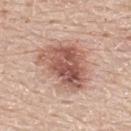Captured during whole-body skin photography for melanoma surveillance; the lesion was not biopsied.
Cropped from a total-body skin-imaging series; the visible field is about 15 mm.
The patient is a male aged around 60.
Automated tile analysis of the lesion measured an area of roughly 22 mm², an outline eccentricity of about 0.7 (0 = round, 1 = elongated), and two-axis asymmetry of about 0.3. And it measured a nevus-likeness score of about 85/100 and a lesion-detection confidence of about 100/100.
From the upper back.